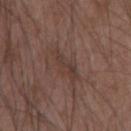| key | value |
|---|---|
| follow-up | total-body-photography surveillance lesion; no biopsy |
| lesion size | about 4.5 mm |
| acquisition | 15 mm crop, total-body photography |
| patient | male, approximately 50 years of age |
| site | the left upper arm |
| illumination | white-light |
| automated lesion analysis | an area of roughly 6.5 mm², an outline eccentricity of about 0.9 (0 = round, 1 = elongated), and a symmetry-axis asymmetry near 0.3; a border-irregularity index near 4.5/10, a within-lesion color-variation index near 2.5/10, and a peripheral color-asymmetry measure near 1 |